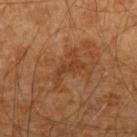* site: the left forearm
* patient: male, aged approximately 60
* acquisition: ~15 mm tile from a whole-body skin photo